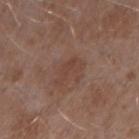biopsy status: imaged on a skin check; not biopsied | tile lighting: white-light illumination | size: ~3.5 mm (longest diameter) | location: the right upper arm | acquisition: 15 mm crop, total-body photography | subject: male, aged 53–57 | automated lesion analysis: an area of roughly 4 mm², an outline eccentricity of about 0.9 (0 = round, 1 = elongated), and two-axis asymmetry of about 0.5; an average lesion color of about L≈42 a*≈19 b*≈25 (CIELAB), roughly 5 lightness units darker than nearby skin, and a normalized lesion–skin contrast near 5; border irregularity of about 6 on a 0–10 scale, internal color variation of about 2 on a 0–10 scale, and peripheral color asymmetry of about 0.5.